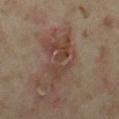Recorded during total-body skin imaging; not selected for excision or biopsy. This image is a 15 mm lesion crop taken from a total-body photograph. The patient is a female in their mid-30s. On the left thigh. Captured under cross-polarized illumination.